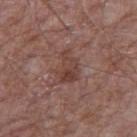This lesion was catalogued during total-body skin photography and was not selected for biopsy. The lesion is on the right upper arm. A male patient, in their mid- to late 60s. An algorithmic analysis of the crop reported a lesion color around L≈41 a*≈20 b*≈23 in CIELAB, roughly 8 lightness units darker than nearby skin, and a normalized lesion–skin contrast near 6.5. It also reported a border-irregularity rating of about 4.5/10, a color-variation rating of about 5.5/10, and a peripheral color-asymmetry measure near 2. The analysis additionally found a detector confidence of about 100 out of 100 that the crop contains a lesion. A roughly 15 mm field-of-view crop from a total-body skin photograph. Longest diameter approximately 4 mm. This is a white-light tile.